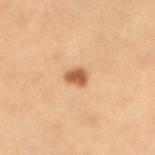Impression:
Recorded during total-body skin imaging; not selected for excision or biopsy.
Acquisition and patient details:
Automated tile analysis of the lesion measured a border-irregularity index near 2/10, a within-lesion color-variation index near 2/10, and a peripheral color-asymmetry measure near 0.5. This image is a 15 mm lesion crop taken from a total-body photograph. About 2.5 mm across. The subject is a male roughly 65 years of age. This is a cross-polarized tile. Located on the right forearm.